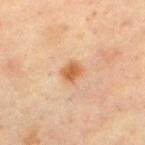biopsy_status: not biopsied; imaged during a skin examination
image:
  source: total-body photography crop
  field_of_view_mm: 15
patient:
  sex: male
  age_approx: 45
lighting: cross-polarized
site: upper back
lesion_size:
  long_diameter_mm_approx: 2.5